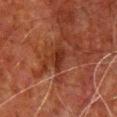| key | value |
|---|---|
| follow-up | imaged on a skin check; not biopsied |
| subject | male, in their 60s |
| lighting | cross-polarized |
| site | the chest |
| image source | 15 mm crop, total-body photography |
| diameter | ~3 mm (longest diameter) |